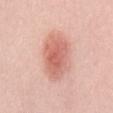biopsy status: imaged on a skin check; not biopsied | illumination: white-light | subject: male, about 25 years old | body site: the back | image source: 15 mm crop, total-body photography | diameter: about 6 mm.